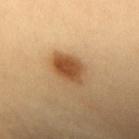Clinical impression:
Part of a total-body skin-imaging series; this lesion was reviewed on a skin check and was not flagged for biopsy.
Background:
A female patient, about 40 years old. The lesion is located on the upper back. The lesion's longest dimension is about 5 mm. Imaged with cross-polarized lighting. A 15 mm crop from a total-body photograph taken for skin-cancer surveillance.